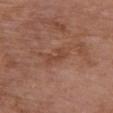Clinical impression:
Recorded during total-body skin imaging; not selected for excision or biopsy.
Clinical summary:
Automated tile analysis of the lesion measured an eccentricity of roughly 0.9 and two-axis asymmetry of about 0.45. The software also gave an average lesion color of about L≈44 a*≈23 b*≈30 (CIELAB) and a lesion-to-skin contrast of about 5.5 (normalized; higher = more distinct). It also reported a border-irregularity rating of about 5.5/10, a color-variation rating of about 0/10, and peripheral color asymmetry of about 0. It also reported an automated nevus-likeness rating near 0 out of 100 and a lesion-detection confidence of about 100/100. A 15 mm close-up tile from a total-body photography series done for melanoma screening. Longest diameter approximately 3 mm. The tile uses white-light illumination. The patient is a female aged approximately 80. The lesion is on the chest.